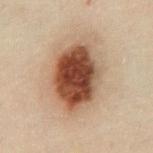follow-up=no biopsy performed (imaged during a skin exam)